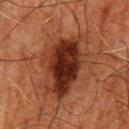Part of a total-body skin-imaging series; this lesion was reviewed on a skin check and was not flagged for biopsy.
A male patient aged approximately 80.
The lesion is located on the mid back.
A close-up tile cropped from a whole-body skin photograph, about 15 mm across.
Measured at roughly 8.5 mm in maximum diameter.
The tile uses cross-polarized illumination.